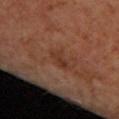Q: Is there a histopathology result?
A: catalogued during a skin exam; not biopsied
Q: Patient demographics?
A: female, aged around 40
Q: Where on the body is the lesion?
A: the upper back
Q: What kind of image is this?
A: total-body-photography crop, ~15 mm field of view